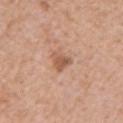Context: This is a white-light tile. A region of skin cropped from a whole-body photographic capture, roughly 15 mm wide. The lesion-visualizer software estimated an average lesion color of about L≈56 a*≈22 b*≈31 (CIELAB), roughly 10 lightness units darker than nearby skin, and a lesion-to-skin contrast of about 7 (normalized; higher = more distinct). The lesion's longest dimension is about 3 mm. The lesion is on the left upper arm. The subject is a female aged 68 to 72.